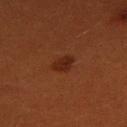Clinical summary:
A female patient about 30 years old. A 15 mm close-up tile from a total-body photography series done for melanoma screening. Longest diameter approximately 3 mm. Automated image analysis of the tile measured a footprint of about 4 mm² and a shape eccentricity near 0.75. And it measured a mean CIELAB color near L≈24 a*≈23 b*≈28, a lesion–skin lightness drop of about 7, and a lesion-to-skin contrast of about 7.5 (normalized; higher = more distinct). And it measured a nevus-likeness score of about 100/100. On the right thigh.